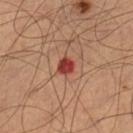<tbp_lesion>
  <biopsy_status>not biopsied; imaged during a skin examination</biopsy_status>
  <image>
    <source>total-body photography crop</source>
    <field_of_view_mm>15</field_of_view_mm>
  </image>
  <automated_metrics>
    <cielab_L>41</cielab_L>
    <cielab_a>33</cielab_a>
    <cielab_b>29</cielab_b>
    <vs_skin_darker_L>14.0</vs_skin_darker_L>
    <vs_skin_contrast_norm>11.0</vs_skin_contrast_norm>
  </automated_metrics>
  <lesion_size>
    <long_diameter_mm_approx>2.0</long_diameter_mm_approx>
  </lesion_size>
  <site>left lower leg</site>
  <patient>
    <sex>male</sex>
    <age_approx>65</age_approx>
  </patient>
  <lighting>cross-polarized</lighting>
</tbp_lesion>